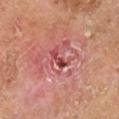Background: A 15 mm crop from a total-body photograph taken for skin-cancer surveillance. A male patient, aged 63 to 67. The tile uses cross-polarized illumination. Located on the leg. The lesion's longest dimension is about 3 mm.